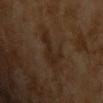Part of a total-body skin-imaging series; this lesion was reviewed on a skin check and was not flagged for biopsy. A lesion tile, about 15 mm wide, cut from a 3D total-body photograph. The tile uses cross-polarized illumination. The subject is a male aged 63 to 67. The total-body-photography lesion software estimated a lesion color around L≈23 a*≈14 b*≈24 in CIELAB and a lesion–skin lightness drop of about 5.